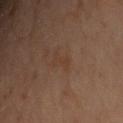Clinical impression:
Recorded during total-body skin imaging; not selected for excision or biopsy.
Clinical summary:
The tile uses cross-polarized illumination. The patient is a male roughly 30 years of age. A close-up tile cropped from a whole-body skin photograph, about 15 mm across. Located on the chest. The total-body-photography lesion software estimated roughly 3 lightness units darker than nearby skin and a normalized lesion–skin contrast near 4.5. And it measured a border-irregularity rating of about 5/10 and radial color variation of about 0. And it measured a classifier nevus-likeness of about 0/100. Longest diameter approximately 3 mm.